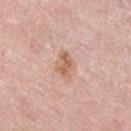Impression: The lesion was tiled from a total-body skin photograph and was not biopsied.